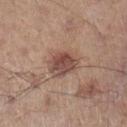workup — catalogued during a skin exam; not biopsied | automated metrics — an area of roughly 9 mm², an outline eccentricity of about 0.6 (0 = round, 1 = elongated), and a shape-asymmetry score of about 0.25 (0 = symmetric); about 11 CIELAB-L* units darker than the surrounding skin; internal color variation of about 5 on a 0–10 scale | tile lighting — white-light illumination | subject — male, approximately 60 years of age | body site — the left lower leg | lesion diameter — ~4 mm (longest diameter) | image source — ~15 mm tile from a whole-body skin photo.